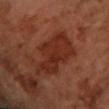The lesion is located on the arm. A 15 mm close-up extracted from a 3D total-body photography capture. The patient is a female aged approximately 70. The total-body-photography lesion software estimated about 8 CIELAB-L* units darker than the surrounding skin. The analysis additionally found a border-irregularity index near 4.5/10, a within-lesion color-variation index near 3/10, and a peripheral color-asymmetry measure near 1. Longest diameter approximately 7 mm.